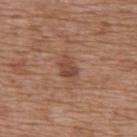The lesion was photographed on a routine skin check and not biopsied; there is no pathology result. Automated image analysis of the tile measured a mean CIELAB color near L≈45 a*≈22 b*≈29, a lesion–skin lightness drop of about 9, and a lesion-to-skin contrast of about 7 (normalized; higher = more distinct). And it measured a border-irregularity rating of about 3/10, a color-variation rating of about 3/10, and peripheral color asymmetry of about 1. Captured under white-light illumination. A 15 mm crop from a total-body photograph taken for skin-cancer surveillance. Located on the upper back. The patient is a female aged around 75.